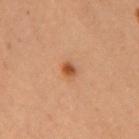Imaged during a routine full-body skin examination; the lesion was not biopsied and no histopathology is available.